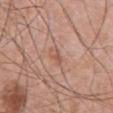Notes:
• biopsy status · catalogued during a skin exam; not biopsied
• image · ~15 mm crop, total-body skin-cancer survey
• image-analysis metrics · a footprint of about 3 mm² and a shape eccentricity near 0.85; a mean CIELAB color near L≈56 a*≈22 b*≈29; a nevus-likeness score of about 0/100
• patient · male, about 70 years old
• anatomic site · the abdomen
• tile lighting · white-light illumination
• diameter · ~2.5 mm (longest diameter)